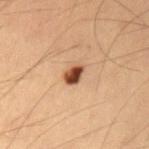<case>
<biopsy_status>not biopsied; imaged during a skin examination</biopsy_status>
<site>left upper arm</site>
<image>
  <source>total-body photography crop</source>
  <field_of_view_mm>15</field_of_view_mm>
</image>
<patient>
  <sex>male</sex>
  <age_approx>35</age_approx>
</patient>
<lesion_size>
  <long_diameter_mm_approx>2.5</long_diameter_mm_approx>
</lesion_size>
<lighting>cross-polarized</lighting>
</case>